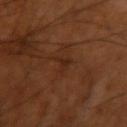• workup · no biopsy performed (imaged during a skin exam)
• image source · total-body-photography crop, ~15 mm field of view
• TBP lesion metrics · an area of roughly 2.5 mm², a shape eccentricity near 0.85, and a symmetry-axis asymmetry near 0.65; roughly 5 lightness units darker than nearby skin and a normalized border contrast of about 5.5; border irregularity of about 7 on a 0–10 scale, a within-lesion color-variation index near 0/10, and radial color variation of about 0
• patient · male, in their 70s
• lighting · cross-polarized
• anatomic site · the arm